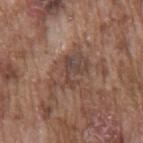This lesion was catalogued during total-body skin photography and was not selected for biopsy. A male patient, aged approximately 75. On the back. Imaged with white-light lighting. A lesion tile, about 15 mm wide, cut from a 3D total-body photograph.